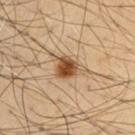workup: imaged on a skin check; not biopsied
image source: 15 mm crop, total-body photography
body site: the front of the torso
automated lesion analysis: a lesion area of about 7 mm², an outline eccentricity of about 0.55 (0 = round, 1 = elongated), and a shape-asymmetry score of about 0.35 (0 = symmetric); border irregularity of about 3.5 on a 0–10 scale and internal color variation of about 6.5 on a 0–10 scale; a classifier nevus-likeness of about 100/100
illumination: cross-polarized
subject: male, aged 53 to 57
lesion diameter: ~3.5 mm (longest diameter)